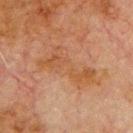Clinical impression: The lesion was photographed on a routine skin check and not biopsied; there is no pathology result. Clinical summary: The lesion is located on the chest. Measured at roughly 7 mm in maximum diameter. A 15 mm close-up tile from a total-body photography series done for melanoma screening. Captured under cross-polarized illumination. A male patient, about 80 years old.